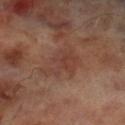The lesion was photographed on a routine skin check and not biopsied; there is no pathology result. The lesion is on the leg. A 15 mm close-up extracted from a 3D total-body photography capture. A male patient aged 68 to 72.